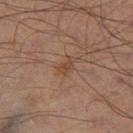Impression:
The lesion was photographed on a routine skin check and not biopsied; there is no pathology result.
Background:
The lesion is located on the right thigh. The patient is a male in their 70s. A lesion tile, about 15 mm wide, cut from a 3D total-body photograph.